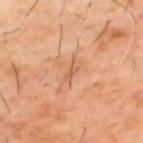The lesion was tiled from a total-body skin photograph and was not biopsied. The recorded lesion diameter is about 2.5 mm. The total-body-photography lesion software estimated a footprint of about 2.5 mm², an eccentricity of roughly 0.9, and two-axis asymmetry of about 0.4. It also reported an average lesion color of about L≈56 a*≈22 b*≈34 (CIELAB), a lesion–skin lightness drop of about 8, and a normalized border contrast of about 5. And it measured a border-irregularity index near 4.5/10. The analysis additionally found an automated nevus-likeness rating near 0 out of 100 and a detector confidence of about 95 out of 100 that the crop contains a lesion. A region of skin cropped from a whole-body photographic capture, roughly 15 mm wide. A male patient, in their 60s. On the back. The tile uses cross-polarized illumination.